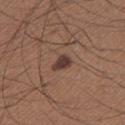About 2.5 mm across.
Cropped from a whole-body photographic skin survey; the tile spans about 15 mm.
Automated image analysis of the tile measured a border-irregularity rating of about 2.5/10 and a within-lesion color-variation index near 1.5/10.
The subject is a male aged around 65.
On the left lower leg.